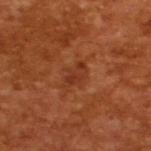Part of a total-body skin-imaging series; this lesion was reviewed on a skin check and was not flagged for biopsy.
Automated tile analysis of the lesion measured a lesion area of about 5.5 mm² and an outline eccentricity of about 0.8 (0 = round, 1 = elongated). It also reported a lesion color around L≈35 a*≈28 b*≈34 in CIELAB, a lesion–skin lightness drop of about 6, and a normalized lesion–skin contrast near 5.5. And it measured a border-irregularity rating of about 3.5/10, a within-lesion color-variation index near 2.5/10, and a peripheral color-asymmetry measure near 1.
This image is a 15 mm lesion crop taken from a total-body photograph.
This is a cross-polarized tile.
A male patient, aged approximately 65.